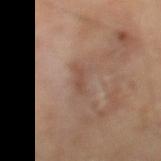Clinical impression: Part of a total-body skin-imaging series; this lesion was reviewed on a skin check and was not flagged for biopsy. Context: The subject is a male aged 63–67. A region of skin cropped from a whole-body photographic capture, roughly 15 mm wide. The total-body-photography lesion software estimated an area of roughly 3 mm², an eccentricity of roughly 0.9, and two-axis asymmetry of about 0.2. The analysis additionally found an average lesion color of about L≈49 a*≈18 b*≈27 (CIELAB), about 7 CIELAB-L* units darker than the surrounding skin, and a normalized lesion–skin contrast near 6. It also reported border irregularity of about 3 on a 0–10 scale and radial color variation of about 0. This is a cross-polarized tile. From the left lower leg.